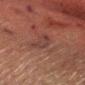Case summary:
– biopsy status · catalogued during a skin exam; not biopsied
– subject · male, in their mid-70s
– image-analysis metrics · a lesion area of about 5.5 mm² and an eccentricity of roughly 0.9; an average lesion color of about L≈32 a*≈19 b*≈20 (CIELAB), a lesion–skin lightness drop of about 5, and a normalized lesion–skin contrast near 5.5; a nevus-likeness score of about 0/100 and lesion-presence confidence of about 80/100
– tile lighting · cross-polarized
– image source · ~15 mm tile from a whole-body skin photo
– location · the head or neck